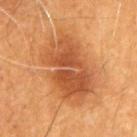Case summary:
• biopsy status · imaged on a skin check; not biopsied
• acquisition · ~15 mm crop, total-body skin-cancer survey
• patient · male, about 60 years old
• location · the arm
• automated lesion analysis · a mean CIELAB color near L≈49 a*≈26 b*≈38, a lesion–skin lightness drop of about 11, and a normalized lesion–skin contrast near 7.5; a nevus-likeness score of about 95/100
• lesion diameter · about 8.5 mm